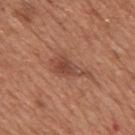biopsy_status: not biopsied; imaged during a skin examination
patient:
  sex: male
  age_approx: 65
site: back
lesion_size:
  long_diameter_mm_approx: 5.0
image:
  source: total-body photography crop
  field_of_view_mm: 15